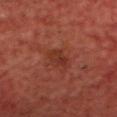<record>
<biopsy_status>not biopsied; imaged during a skin examination</biopsy_status>
</record>